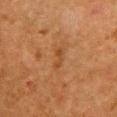biopsy status — no biopsy performed (imaged during a skin exam)
automated metrics — a lesion color around L≈38 a*≈20 b*≈34 in CIELAB, about 6 CIELAB-L* units darker than the surrounding skin, and a normalized lesion–skin contrast near 5.5; a nevus-likeness score of about 0/100 and a lesion-detection confidence of about 100/100
acquisition — ~15 mm tile from a whole-body skin photo
illumination — cross-polarized
diameter — about 3 mm
subject — female, about 50 years old
site — the chest